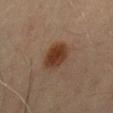Part of a total-body skin-imaging series; this lesion was reviewed on a skin check and was not flagged for biopsy.
The patient is a male about 70 years old.
Imaged with cross-polarized lighting.
A 15 mm crop from a total-body photograph taken for skin-cancer surveillance.
The lesion is located on the lower back.
Automated image analysis of the tile measured a border-irregularity rating of about 1.5/10 and internal color variation of about 2.5 on a 0–10 scale. It also reported a nevus-likeness score of about 100/100 and a lesion-detection confidence of about 100/100.